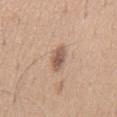<record>
<biopsy_status>not biopsied; imaged during a skin examination</biopsy_status>
<lighting>white-light</lighting>
<lesion_size>
  <long_diameter_mm_approx>3.5</long_diameter_mm_approx>
</lesion_size>
<image>
  <source>total-body photography crop</source>
  <field_of_view_mm>15</field_of_view_mm>
</image>
<patient>
  <sex>male</sex>
  <age_approx>65</age_approx>
</patient>
<automated_metrics>
  <border_irregularity_0_10>2.0</border_irregularity_0_10>
  <color_variation_0_10>3.0</color_variation_0_10>
  <peripheral_color_asymmetry>1.0</peripheral_color_asymmetry>
  <nevus_likeness_0_100>90</nevus_likeness_0_100>
</automated_metrics>
<site>chest</site>
</record>